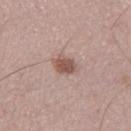Clinical impression:
Captured during whole-body skin photography for melanoma surveillance; the lesion was not biopsied.
Acquisition and patient details:
A male subject in their 70s. The lesion's longest dimension is about 2.5 mm. A 15 mm close-up extracted from a 3D total-body photography capture. The lesion is on the left thigh. This is a white-light tile.